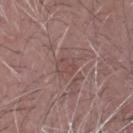Impression: Captured during whole-body skin photography for melanoma surveillance; the lesion was not biopsied. Context: The lesion is located on the head or neck. A roughly 15 mm field-of-view crop from a total-body skin photograph. A male subject in their mid- to late 70s. Approximately 2.5 mm at its widest. Captured under white-light illumination. Automated image analysis of the tile measured an average lesion color of about L≈47 a*≈19 b*≈21 (CIELAB), roughly 6 lightness units darker than nearby skin, and a normalized lesion–skin contrast near 4.5. And it measured an automated nevus-likeness rating near 0 out of 100 and a detector confidence of about 80 out of 100 that the crop contains a lesion.